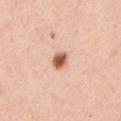Q: Was this lesion biopsied?
A: total-body-photography surveillance lesion; no biopsy
Q: Where on the body is the lesion?
A: the arm
Q: Patient demographics?
A: male, about 50 years old
Q: What is the imaging modality?
A: ~15 mm crop, total-body skin-cancer survey